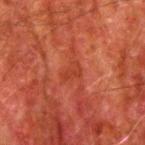biopsy_status: not biopsied; imaged during a skin examination
lighting: cross-polarized
patient:
  sex: male
  age_approx: 80
image:
  source: total-body photography crop
  field_of_view_mm: 15
site: right upper arm
lesion_size:
  long_diameter_mm_approx: 2.5